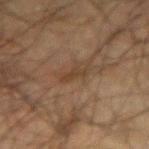Findings:
• follow-up — no biopsy performed (imaged during a skin exam)
• body site — the arm
• patient — male, about 70 years old
• image source — total-body-photography crop, ~15 mm field of view
• lighting — cross-polarized
• lesion diameter — about 2.5 mm
• automated lesion analysis — a symmetry-axis asymmetry near 0.45; border irregularity of about 5 on a 0–10 scale; lesion-presence confidence of about 100/100